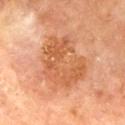Q: Was this lesion biopsied?
A: catalogued during a skin exam; not biopsied
Q: Patient demographics?
A: male, approximately 70 years of age
Q: What lighting was used for the tile?
A: cross-polarized
Q: Lesion location?
A: the mid back
Q: What kind of image is this?
A: 15 mm crop, total-body photography
Q: Lesion size?
A: about 6.5 mm